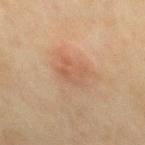follow-up = catalogued during a skin exam; not biopsied | lesion diameter = ≈4 mm | lighting = cross-polarized illumination | subject = male, aged approximately 45 | body site = the mid back | image source = 15 mm crop, total-body photography.